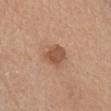Impression: Recorded during total-body skin imaging; not selected for excision or biopsy. Background: This is a white-light tile. A roughly 15 mm field-of-view crop from a total-body skin photograph. Located on the chest. Automated tile analysis of the lesion measured an average lesion color of about L≈52 a*≈21 b*≈32 (CIELAB), roughly 11 lightness units darker than nearby skin, and a normalized lesion–skin contrast near 8. And it measured border irregularity of about 2 on a 0–10 scale and radial color variation of about 1.5. The subject is a male roughly 60 years of age.